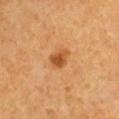Clinical impression:
The lesion was tiled from a total-body skin photograph and was not biopsied.
Image and clinical context:
Cropped from a whole-body photographic skin survey; the tile spans about 15 mm. The tile uses cross-polarized illumination. The lesion's longest dimension is about 2.5 mm. The total-body-photography lesion software estimated a footprint of about 4.5 mm². The software also gave a lesion color around L≈50 a*≈26 b*≈42 in CIELAB and about 11 CIELAB-L* units darker than the surrounding skin. The analysis additionally found border irregularity of about 2.5 on a 0–10 scale, a color-variation rating of about 2.5/10, and peripheral color asymmetry of about 1. It also reported an automated nevus-likeness rating near 95 out of 100 and a detector confidence of about 100 out of 100 that the crop contains a lesion. From the left upper arm. A male subject, aged 58 to 62.